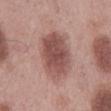site: mid back
lesion_size:
  long_diameter_mm_approx: 7.5
image:
  source: total-body photography crop
  field_of_view_mm: 15
lighting: white-light
patient:
  sex: male
  age_approx: 55